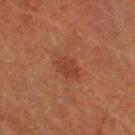Case summary:
* patient · male, in their mid-70s
* image-analysis metrics · a border-irregularity rating of about 2/10, a color-variation rating of about 2/10, and radial color variation of about 0.5; a detector confidence of about 100 out of 100 that the crop contains a lesion
* image · ~15 mm crop, total-body skin-cancer survey
* illumination · cross-polarized
* size · ~3 mm (longest diameter)
* site · the right forearm